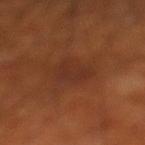biopsy status: imaged on a skin check; not biopsied
subject: male, approximately 70 years of age
tile lighting: cross-polarized illumination
body site: the left lower leg
imaging modality: total-body-photography crop, ~15 mm field of view
image-analysis metrics: an eccentricity of roughly 0.6 and a shape-asymmetry score of about 0.3 (0 = symmetric); a mean CIELAB color near L≈30 a*≈23 b*≈28 and a normalized border contrast of about 5.5